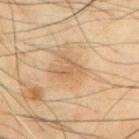Recorded during total-body skin imaging; not selected for excision or biopsy. A 15 mm close-up tile from a total-body photography series done for melanoma screening. The lesion is on the chest. Imaged with cross-polarized lighting. Approximately 3 mm at its widest. The subject is a male in their mid- to late 50s.